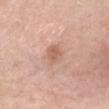Part of a total-body skin-imaging series; this lesion was reviewed on a skin check and was not flagged for biopsy. A roughly 15 mm field-of-view crop from a total-body skin photograph. A female patient, aged 63 to 67. Captured under white-light illumination. Longest diameter approximately 2.5 mm. Located on the upper back.